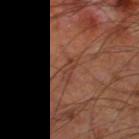Clinical impression:
Part of a total-body skin-imaging series; this lesion was reviewed on a skin check and was not flagged for biopsy.
Background:
The lesion is on the left thigh. The lesion-visualizer software estimated a lesion area of about 3.5 mm², an outline eccentricity of about 0.85 (0 = round, 1 = elongated), and a shape-asymmetry score of about 0.35 (0 = symmetric). And it measured a mean CIELAB color near L≈36 a*≈21 b*≈25 and a lesion-to-skin contrast of about 5 (normalized; higher = more distinct). The software also gave an automated nevus-likeness rating near 0 out of 100 and a detector confidence of about 95 out of 100 that the crop contains a lesion. Cropped from a total-body skin-imaging series; the visible field is about 15 mm. The subject is a male in their 60s. This is a cross-polarized tile.